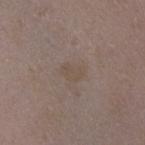Recorded during total-body skin imaging; not selected for excision or biopsy. The lesion is on the chest. The lesion-visualizer software estimated a mean CIELAB color near L≈48 a*≈12 b*≈23 and a lesion-to-skin contrast of about 4.5 (normalized; higher = more distinct). The analysis additionally found an automated nevus-likeness rating near 0 out of 100 and lesion-presence confidence of about 100/100. Measured at roughly 2.5 mm in maximum diameter. A lesion tile, about 15 mm wide, cut from a 3D total-body photograph. Captured under white-light illumination. A female subject, roughly 35 years of age.